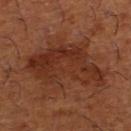This image is a 15 mm lesion crop taken from a total-body photograph.
A male patient, about 65 years old.
An algorithmic analysis of the crop reported lesion-presence confidence of about 100/100.
The tile uses cross-polarized illumination.
Approximately 9 mm at its widest.
The lesion is on the right lower leg.